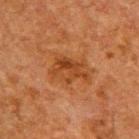Assessment:
No biopsy was performed on this lesion — it was imaged during a full skin examination and was not determined to be concerning.
Clinical summary:
From the upper back. The recorded lesion diameter is about 4.5 mm. A roughly 15 mm field-of-view crop from a total-body skin photograph. The subject is a male aged approximately 60.